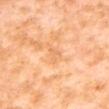Image and clinical context: A female subject, in their mid- to late 50s. On the upper back. Approximately 2.5 mm at its widest. This is a cross-polarized tile. A close-up tile cropped from a whole-body skin photograph, about 15 mm across.